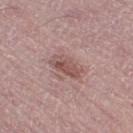A male patient, aged 48–52.
Located on the right thigh.
A 15 mm crop from a total-body photograph taken for skin-cancer surveillance.